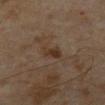biopsy_status: not biopsied; imaged during a skin examination
lighting: cross-polarized
image:
  source: total-body photography crop
  field_of_view_mm: 15
patient:
  sex: male
  age_approx: 65